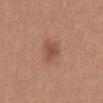Q: Was this lesion biopsied?
A: imaged on a skin check; not biopsied
Q: How was this image acquired?
A: total-body-photography crop, ~15 mm field of view
Q: What did automated image analysis measure?
A: an area of roughly 5.5 mm², an eccentricity of roughly 0.65, and two-axis asymmetry of about 0.3; a border-irregularity index near 2.5/10 and a color-variation rating of about 2.5/10
Q: What lighting was used for the tile?
A: white-light
Q: How large is the lesion?
A: ~3 mm (longest diameter)
Q: What are the patient's age and sex?
A: female, roughly 40 years of age
Q: What is the anatomic site?
A: the abdomen